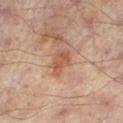This lesion was catalogued during total-body skin photography and was not selected for biopsy.
A male patient, roughly 65 years of age.
The lesion is on the left lower leg.
The lesion's longest dimension is about 3 mm.
A 15 mm crop from a total-body photograph taken for skin-cancer surveillance.
Automated image analysis of the tile measured a lesion area of about 5 mm², a shape eccentricity near 0.75, and a shape-asymmetry score of about 0.25 (0 = symmetric). And it measured internal color variation of about 2.5 on a 0–10 scale. The analysis additionally found a lesion-detection confidence of about 100/100.
Imaged with cross-polarized lighting.